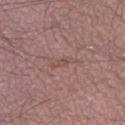| feature | finding |
|---|---|
| notes | imaged on a skin check; not biopsied |
| site | the left lower leg |
| TBP lesion metrics | a footprint of about 2.5 mm² and two-axis asymmetry of about 0.25; a border-irregularity index near 3.5/10 and a color-variation rating of about 0/10; an automated nevus-likeness rating near 0 out of 100 and lesion-presence confidence of about 90/100 |
| patient | male, in their mid-20s |
| acquisition | total-body-photography crop, ~15 mm field of view |